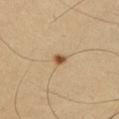{
  "biopsy_status": "not biopsied; imaged during a skin examination",
  "image": {
    "source": "total-body photography crop",
    "field_of_view_mm": 15
  },
  "site": "right upper arm",
  "lesion_size": {
    "long_diameter_mm_approx": 1.5
  },
  "automated_metrics": {
    "area_mm2_approx": 2.0,
    "vs_skin_darker_L": 14.0,
    "vs_skin_contrast_norm": 9.5,
    "nevus_likeness_0_100": 95
  },
  "patient": {
    "sex": "male",
    "age_approx": 40
  }
}